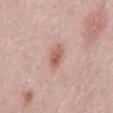Imaged during a routine full-body skin examination; the lesion was not biopsied and no histopathology is available. This image is a 15 mm lesion crop taken from a total-body photograph. The subject is a male aged approximately 70. From the front of the torso.